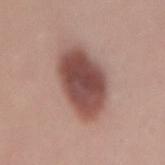Part of a total-body skin-imaging series; this lesion was reviewed on a skin check and was not flagged for biopsy. The total-body-photography lesion software estimated border irregularity of about 1.5 on a 0–10 scale and internal color variation of about 4.5 on a 0–10 scale. From the lower back. Approximately 7 mm at its widest. This is a white-light tile. A roughly 15 mm field-of-view crop from a total-body skin photograph. A female subject in their mid- to late 20s.